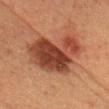Impression:
Recorded during total-body skin imaging; not selected for excision or biopsy.
Image and clinical context:
Located on the head or neck. This is a cross-polarized tile. Cropped from a total-body skin-imaging series; the visible field is about 15 mm. The recorded lesion diameter is about 8 mm. A female patient in their mid-50s. Automated tile analysis of the lesion measured a border-irregularity rating of about 4.5/10. And it measured an automated nevus-likeness rating near 90 out of 100 and a detector confidence of about 100 out of 100 that the crop contains a lesion.